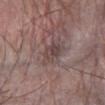Assessment: The lesion was tiled from a total-body skin photograph and was not biopsied. Context: This is a white-light tile. A roughly 15 mm field-of-view crop from a total-body skin photograph. An algorithmic analysis of the crop reported a within-lesion color-variation index near 4/10 and peripheral color asymmetry of about 1.5. Located on the arm. A male patient, about 65 years old. Approximately 4.5 mm at its widest.